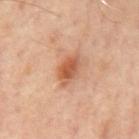{"biopsy_status": "not biopsied; imaged during a skin examination", "lighting": "cross-polarized", "image": {"source": "total-body photography crop", "field_of_view_mm": 15}, "automated_metrics": {"cielab_L": 54, "cielab_a": 25, "cielab_b": 34, "vs_skin_darker_L": 12.0, "vs_skin_contrast_norm": 8.5, "border_irregularity_0_10": 2.5, "peripheral_color_asymmetry": 1.5, "nevus_likeness_0_100": 80, "lesion_detection_confidence_0_100": 100}, "lesion_size": {"long_diameter_mm_approx": 4.0}, "patient": {"sex": "male", "age_approx": 65}, "site": "back"}